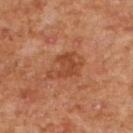Assessment:
Part of a total-body skin-imaging series; this lesion was reviewed on a skin check and was not flagged for biopsy.
Image and clinical context:
The lesion's longest dimension is about 4.5 mm. The subject is a female aged 58 to 62. The lesion is on the back. Imaged with cross-polarized lighting. A close-up tile cropped from a whole-body skin photograph, about 15 mm across.